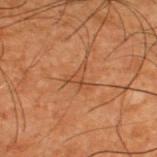This lesion was catalogued during total-body skin photography and was not selected for biopsy.
The tile uses cross-polarized illumination.
A region of skin cropped from a whole-body photographic capture, roughly 15 mm wide.
Approximately 2.5 mm at its widest.
A male subject, aged 48–52.
From the upper back.